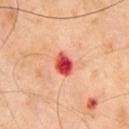biopsy_status: not biopsied; imaged during a skin examination
lighting: cross-polarized
automated_metrics:
  area_mm2_approx: 5.0
  eccentricity: 0.7
  shape_asymmetry: 0.2
patient:
  sex: male
  age_approx: 65
image:
  source: total-body photography crop
  field_of_view_mm: 15